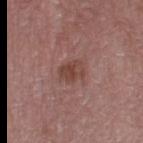This lesion was catalogued during total-body skin photography and was not selected for biopsy.
The subject is a female aged around 70.
Measured at roughly 3 mm in maximum diameter.
An algorithmic analysis of the crop reported an area of roughly 6 mm², an outline eccentricity of about 0.65 (0 = round, 1 = elongated), and two-axis asymmetry of about 0.2. The analysis additionally found an average lesion color of about L≈43 a*≈21 b*≈23 (CIELAB) and about 8 CIELAB-L* units darker than the surrounding skin. The analysis additionally found a nevus-likeness score of about 20/100.
Captured under white-light illumination.
The lesion is on the left thigh.
A region of skin cropped from a whole-body photographic capture, roughly 15 mm wide.